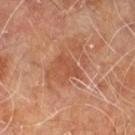<lesion>
<biopsy_status>not biopsied; imaged during a skin examination</biopsy_status>
<lesion_size>
  <long_diameter_mm_approx>3.5</long_diameter_mm_approx>
</lesion_size>
<lighting>cross-polarized</lighting>
<patient>
  <sex>male</sex>
  <age_approx>60</age_approx>
</patient>
<image>
  <source>total-body photography crop</source>
  <field_of_view_mm>15</field_of_view_mm>
</image>
<site>leg</site>
<automated_metrics>
  <area_mm2_approx>5.0</area_mm2_approx>
  <shape_asymmetry>0.25</shape_asymmetry>
  <cielab_L>48</cielab_L>
  <cielab_a>26</cielab_a>
  <cielab_b>32</cielab_b>
  <vs_skin_darker_L>7.0</vs_skin_darker_L>
  <vs_skin_contrast_norm>5.0</vs_skin_contrast_norm>
  <nevus_likeness_0_100>0</nevus_likeness_0_100>
</automated_metrics>
</lesion>